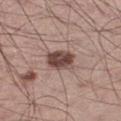notes = imaged on a skin check; not biopsied | location = the leg | subject = male, aged 53–57 | image = ~15 mm crop, total-body skin-cancer survey | lesion size = ≈3.5 mm | lighting = white-light | automated lesion analysis = a footprint of about 8 mm²; a mean CIELAB color near L≈45 a*≈18 b*≈22; border irregularity of about 1.5 on a 0–10 scale, a color-variation rating of about 5/10, and a peripheral color-asymmetry measure near 2.